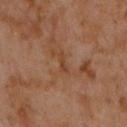Imaged during a routine full-body skin examination; the lesion was not biopsied and no histopathology is available.
The patient is a male roughly 60 years of age.
Approximately 2.5 mm at its widest.
This is a cross-polarized tile.
On the chest.
A 15 mm crop from a total-body photograph taken for skin-cancer surveillance.